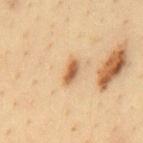The lesion was tiled from a total-body skin photograph and was not biopsied.
Approximately 3 mm at its widest.
A region of skin cropped from a whole-body photographic capture, roughly 15 mm wide.
From the back.
The total-body-photography lesion software estimated an area of roughly 4 mm² and a shape-asymmetry score of about 0.2 (0 = symmetric). The software also gave a lesion–skin lightness drop of about 13 and a normalized border contrast of about 8.5. The software also gave border irregularity of about 2 on a 0–10 scale, internal color variation of about 3 on a 0–10 scale, and a peripheral color-asymmetry measure near 1.
The patient is a male in their mid-30s.